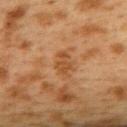{"biopsy_status": "not biopsied; imaged during a skin examination", "lesion_size": {"long_diameter_mm_approx": 2.5}, "patient": {"sex": "female", "age_approx": 40}, "image": {"source": "total-body photography crop", "field_of_view_mm": 15}, "automated_metrics": {"vs_skin_contrast_norm": 6.5}, "site": "upper back", "lighting": "cross-polarized"}